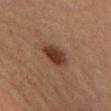| feature | finding |
|---|---|
| biopsy status | no biopsy performed (imaged during a skin exam) |
| tile lighting | cross-polarized |
| subject | female, aged around 20 |
| body site | the left lower leg |
| imaging modality | 15 mm crop, total-body photography |
| size | about 3.5 mm |